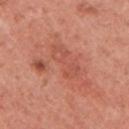| field | value |
|---|---|
| workup | imaged on a skin check; not biopsied |
| acquisition | total-body-photography crop, ~15 mm field of view |
| body site | the arm |
| patient | female, in their 50s |